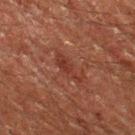{
  "biopsy_status": "not biopsied; imaged during a skin examination",
  "patient": {
    "sex": "male",
    "age_approx": 60
  },
  "image": {
    "source": "total-body photography crop",
    "field_of_view_mm": 15
  },
  "site": "left thigh"
}